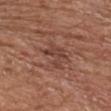Clinical impression:
Part of a total-body skin-imaging series; this lesion was reviewed on a skin check and was not flagged for biopsy.
Acquisition and patient details:
This is a white-light tile. On the front of the torso. A roughly 15 mm field-of-view crop from a total-body skin photograph. A female patient about 75 years old. Measured at roughly 3.5 mm in maximum diameter. Automated tile analysis of the lesion measured a lesion area of about 5.5 mm² and a shape eccentricity near 0.7. It also reported border irregularity of about 6.5 on a 0–10 scale, a within-lesion color-variation index near 1.5/10, and peripheral color asymmetry of about 0.5. It also reported a classifier nevus-likeness of about 0/100.